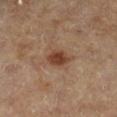This lesion was catalogued during total-body skin photography and was not selected for biopsy. A 15 mm crop from a total-body photograph taken for skin-cancer surveillance. A male patient, aged around 85 or older. Imaged with cross-polarized lighting. The lesion is located on the right lower leg.